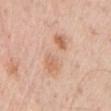No biopsy was performed on this lesion — it was imaged during a full skin examination and was not determined to be concerning. The recorded lesion diameter is about 7 mm. A close-up tile cropped from a whole-body skin photograph, about 15 mm across. Automated image analysis of the tile measured a lesion area of about 14 mm² and two-axis asymmetry of about 0.35. The analysis additionally found a mean CIELAB color near L≈66 a*≈20 b*≈32, a lesion–skin lightness drop of about 8, and a normalized border contrast of about 5.5. The analysis additionally found a border-irregularity index near 6/10, internal color variation of about 6 on a 0–10 scale, and a peripheral color-asymmetry measure near 2. And it measured an automated nevus-likeness rating near 0 out of 100 and a detector confidence of about 100 out of 100 that the crop contains a lesion. Located on the chest. The patient is a male approximately 75 years of age.